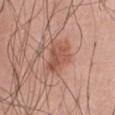Recorded during total-body skin imaging; not selected for excision or biopsy. A male patient aged approximately 55. The lesion-visualizer software estimated an average lesion color of about L≈53 a*≈24 b*≈29 (CIELAB) and about 9 CIELAB-L* units darker than the surrounding skin. And it measured border irregularity of about 4 on a 0–10 scale, a color-variation rating of about 3.5/10, and peripheral color asymmetry of about 1.5. It also reported an automated nevus-likeness rating near 30 out of 100 and a detector confidence of about 100 out of 100 that the crop contains a lesion. This image is a 15 mm lesion crop taken from a total-body photograph. Imaged with white-light lighting. From the chest.